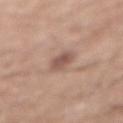follow-up: total-body-photography surveillance lesion; no biopsy
illumination: white-light illumination
image source: ~15 mm crop, total-body skin-cancer survey
body site: the abdomen
subject: male, aged around 70
lesion diameter: ≈3 mm